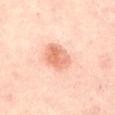The lesion was tiled from a total-body skin photograph and was not biopsied. The recorded lesion diameter is about 3.5 mm. The patient is a female approximately 40 years of age. A 15 mm close-up tile from a total-body photography series done for melanoma screening. The lesion is located on the abdomen. This is a cross-polarized tile.